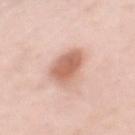Imaged during a routine full-body skin examination; the lesion was not biopsied and no histopathology is available.
From the mid back.
An algorithmic analysis of the crop reported a lesion color around L≈64 a*≈22 b*≈29 in CIELAB and a lesion-to-skin contrast of about 8 (normalized; higher = more distinct).
Measured at roughly 5.5 mm in maximum diameter.
A 15 mm close-up extracted from a 3D total-body photography capture.
A female patient, aged 48 to 52.